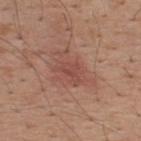  biopsy_status: not biopsied; imaged during a skin examination
  image:
    source: total-body photography crop
    field_of_view_mm: 15
  site: upper back
  patient:
    sex: male
    age_approx: 40
  lighting: white-light